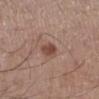No biopsy was performed on this lesion — it was imaged during a full skin examination and was not determined to be concerning.
A male patient, approximately 55 years of age.
This is a white-light tile.
A 15 mm close-up tile from a total-body photography series done for melanoma screening.
The lesion is located on the left lower leg.
Measured at roughly 2.5 mm in maximum diameter.